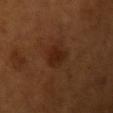Q: Was a biopsy performed?
A: total-body-photography surveillance lesion; no biopsy
Q: Illumination type?
A: cross-polarized
Q: Who is the patient?
A: female, aged 53 to 57
Q: What is the anatomic site?
A: the left upper arm
Q: What kind of image is this?
A: ~15 mm tile from a whole-body skin photo
Q: How large is the lesion?
A: ~3 mm (longest diameter)
Q: Automated lesion metrics?
A: a lesion color around L≈24 a*≈21 b*≈29 in CIELAB, a lesion–skin lightness drop of about 7, and a lesion-to-skin contrast of about 7.5 (normalized; higher = more distinct); a border-irregularity rating of about 3/10 and a color-variation rating of about 1.5/10